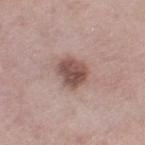| feature | finding |
|---|---|
| notes | catalogued during a skin exam; not biopsied |
| lighting | white-light |
| image | 15 mm crop, total-body photography |
| subject | female, approximately 55 years of age |
| body site | the left thigh |